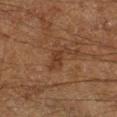Q: Was a biopsy performed?
A: catalogued during a skin exam; not biopsied
Q: What is the lesion's diameter?
A: ~3.5 mm (longest diameter)
Q: Lesion location?
A: the leg
Q: What are the patient's age and sex?
A: male, roughly 60 years of age
Q: Automated lesion metrics?
A: a shape-asymmetry score of about 0.6 (0 = symmetric); an automated nevus-likeness rating near 5 out of 100
Q: Illumination type?
A: cross-polarized
Q: What kind of image is this?
A: total-body-photography crop, ~15 mm field of view